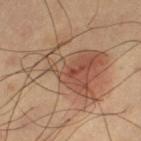| key | value |
|---|---|
| workup | no biopsy performed (imaged during a skin exam) |
| site | the left thigh |
| tile lighting | cross-polarized illumination |
| image | total-body-photography crop, ~15 mm field of view |
| size | ≈9.5 mm |
| TBP lesion metrics | an area of roughly 43 mm² and an eccentricity of roughly 0.75; an average lesion color of about L≈51 a*≈18 b*≈29 (CIELAB) and roughly 9 lightness units darker than nearby skin; a border-irregularity index near 6.5/10 and peripheral color asymmetry of about 3 |
| subject | male, about 60 years old |